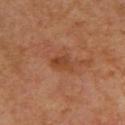workup — catalogued during a skin exam; not biopsied | lesion size — about 3 mm | anatomic site — the upper back | tile lighting — cross-polarized illumination | image source — total-body-photography crop, ~15 mm field of view | subject — female, aged approximately 40.